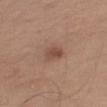The tile uses white-light illumination. A 15 mm close-up tile from a total-body photography series done for melanoma screening. A male patient, in their mid-50s. Approximately 3 mm at its widest. The lesion is located on the left upper arm. An algorithmic analysis of the crop reported a lesion area of about 4 mm², an outline eccentricity of about 0.8 (0 = round, 1 = elongated), and two-axis asymmetry of about 0.2. The software also gave border irregularity of about 2 on a 0–10 scale and peripheral color asymmetry of about 1. The analysis additionally found an automated nevus-likeness rating near 60 out of 100.